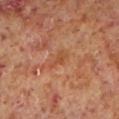Part of a total-body skin-imaging series; this lesion was reviewed on a skin check and was not flagged for biopsy. The lesion's longest dimension is about 2.5 mm. The patient is a male aged 58–62. Located on the left lower leg. This image is a 15 mm lesion crop taken from a total-body photograph.